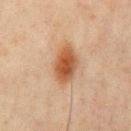biopsy status = catalogued during a skin exam; not biopsied | patient = male, about 45 years old | automated lesion analysis = a border-irregularity rating of about 2/10, a color-variation rating of about 4.5/10, and peripheral color asymmetry of about 1; a nevus-likeness score of about 100/100 and a lesion-detection confidence of about 100/100 | imaging modality = 15 mm crop, total-body photography | anatomic site = the front of the torso.